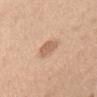illumination = white-light illumination | lesion size = ~3 mm (longest diameter) | imaging modality = ~15 mm crop, total-body skin-cancer survey | location = the head or neck | subject = female, aged around 40 | TBP lesion metrics = roughly 10 lightness units darker than nearby skin and a normalized border contrast of about 6.5.